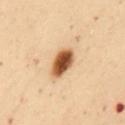The lesion was tiled from a total-body skin photograph and was not biopsied. A 15 mm close-up extracted from a 3D total-body photography capture. The subject is a female aged around 50. The lesion is on the abdomen.